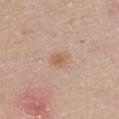Q: Is there a histopathology result?
A: no biopsy performed (imaged during a skin exam)
Q: How large is the lesion?
A: about 2.5 mm
Q: How was this image acquired?
A: 15 mm crop, total-body photography
Q: What is the anatomic site?
A: the chest
Q: Who is the patient?
A: male, in their 60s